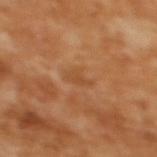Imaged during a routine full-body skin examination; the lesion was not biopsied and no histopathology is available. An algorithmic analysis of the crop reported a footprint of about 3 mm², an outline eccentricity of about 0.8 (0 = round, 1 = elongated), and two-axis asymmetry of about 0.3. And it measured a mean CIELAB color near L≈50 a*≈23 b*≈39 and a lesion-to-skin contrast of about 4.5 (normalized; higher = more distinct). It also reported border irregularity of about 3.5 on a 0–10 scale. And it measured an automated nevus-likeness rating near 0 out of 100 and lesion-presence confidence of about 100/100. A female patient aged 53 to 57. The lesion is on the upper back. About 2.5 mm across. A 15 mm close-up tile from a total-body photography series done for melanoma screening. Imaged with cross-polarized lighting.